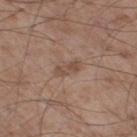Impression:
This lesion was catalogued during total-body skin photography and was not selected for biopsy.
Acquisition and patient details:
From the leg. A 15 mm close-up extracted from a 3D total-body photography capture. Longest diameter approximately 3 mm. The patient is a male approximately 55 years of age. Captured under white-light illumination.